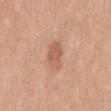This lesion was catalogued during total-body skin photography and was not selected for biopsy.
The tile uses white-light illumination.
On the front of the torso.
A female patient, aged around 35.
The lesion's longest dimension is about 3.5 mm.
A 15 mm crop from a total-body photograph taken for skin-cancer surveillance.